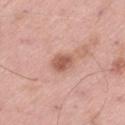* biopsy status · catalogued during a skin exam; not biopsied
* site · the left thigh
* tile lighting · white-light
* image-analysis metrics · an area of roughly 4.5 mm², a shape eccentricity near 0.7, and a symmetry-axis asymmetry near 0.2; an average lesion color of about L≈56 a*≈24 b*≈28 (CIELAB), roughly 12 lightness units darker than nearby skin, and a normalized border contrast of about 8; border irregularity of about 1.5 on a 0–10 scale and a color-variation rating of about 1.5/10; an automated nevus-likeness rating near 70 out of 100 and a detector confidence of about 100 out of 100 that the crop contains a lesion
* imaging modality · total-body-photography crop, ~15 mm field of view
* subject · male, approximately 55 years of age